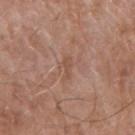anatomic site — the arm
acquisition — ~15 mm tile from a whole-body skin photo
patient — male, aged 73–77
lesion diameter — ~2.5 mm (longest diameter)
tile lighting — white-light illumination
automated metrics — an area of roughly 1.5 mm² and a shape-asymmetry score of about 0.5 (0 = symmetric); an average lesion color of about L≈50 a*≈20 b*≈29 (CIELAB), a lesion–skin lightness drop of about 7, and a lesion-to-skin contrast of about 5.5 (normalized; higher = more distinct); a border-irregularity index near 6/10, a color-variation rating of about 0/10, and radial color variation of about 0